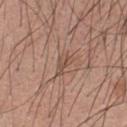This lesion was catalogued during total-body skin photography and was not selected for biopsy. The lesion is on the chest. The recorded lesion diameter is about 3 mm. This image is a 15 mm lesion crop taken from a total-body photograph. The patient is a male aged around 25.